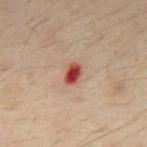A lesion tile, about 15 mm wide, cut from a 3D total-body photograph.
A male subject, aged approximately 50.
From the mid back.